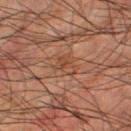Q: Was this lesion biopsied?
A: total-body-photography surveillance lesion; no biopsy
Q: How was this image acquired?
A: total-body-photography crop, ~15 mm field of view
Q: Lesion location?
A: the right thigh
Q: What are the patient's age and sex?
A: male, aged approximately 65